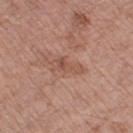  biopsy_status: not biopsied; imaged during a skin examination
  lesion_size:
    long_diameter_mm_approx: 3.5
  image:
    source: total-body photography crop
    field_of_view_mm: 15
  patient:
    sex: female
    age_approx: 75
  lighting: white-light
  site: leg